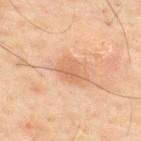biopsy_status: not biopsied; imaged during a skin examination
image:
  source: total-body photography crop
  field_of_view_mm: 15
automated_metrics:
  color_variation_0_10: 2.0
  peripheral_color_asymmetry: 1.0
site: upper back
lighting: cross-polarized
patient:
  sex: male
  age_approx: 60
lesion_size:
  long_diameter_mm_approx: 3.0